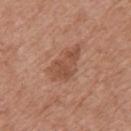{"biopsy_status": "not biopsied; imaged during a skin examination", "image": {"source": "total-body photography crop", "field_of_view_mm": 15}, "patient": {"sex": "male", "age_approx": 80}, "lighting": "white-light", "automated_metrics": {"area_mm2_approx": 8.0, "eccentricity": 0.9, "shape_asymmetry": 0.4, "border_irregularity_0_10": 5.5, "color_variation_0_10": 2.5, "peripheral_color_asymmetry": 1.0, "nevus_likeness_0_100": 15, "lesion_detection_confidence_0_100": 100}, "lesion_size": {"long_diameter_mm_approx": 5.0}, "site": "upper back"}